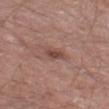Impression: Captured during whole-body skin photography for melanoma surveillance; the lesion was not biopsied. Context: The patient is a male aged 68 to 72. The tile uses white-light illumination. This image is a 15 mm lesion crop taken from a total-body photograph. From the left thigh.